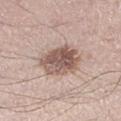The lesion was tiled from a total-body skin photograph and was not biopsied. This image is a 15 mm lesion crop taken from a total-body photograph. The lesion is on the left lower leg. The tile uses white-light illumination. Longest diameter approximately 5.5 mm. A male subject about 35 years old.